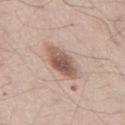Clinical impression:
Part of a total-body skin-imaging series; this lesion was reviewed on a skin check and was not flagged for biopsy.
Acquisition and patient details:
A male patient, approximately 55 years of age. A roughly 15 mm field-of-view crop from a total-body skin photograph. Longest diameter approximately 5 mm. The tile uses white-light illumination. The lesion is on the abdomen.